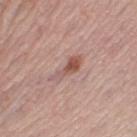<lesion>
<biopsy_status>not biopsied; imaged during a skin examination</biopsy_status>
<image>
  <source>total-body photography crop</source>
  <field_of_view_mm>15</field_of_view_mm>
</image>
<patient>
  <sex>female</sex>
  <age_approx>45</age_approx>
</patient>
<lighting>white-light</lighting>
<automated_metrics>
  <eccentricity>0.95</eccentricity>
  <shape_asymmetry>0.4</shape_asymmetry>
</automated_metrics>
<site>leg</site>
<lesion_size>
  <long_diameter_mm_approx>4.5</long_diameter_mm_approx>
</lesion_size>
</lesion>